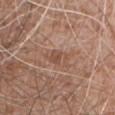Impression: The lesion was tiled from a total-body skin photograph and was not biopsied. Acquisition and patient details: The subject is a male about 60 years old. The tile uses white-light illumination. Located on the lower back. A lesion tile, about 15 mm wide, cut from a 3D total-body photograph. About 2.5 mm across. Automated tile analysis of the lesion measured an average lesion color of about L≈50 a*≈20 b*≈29 (CIELAB), a lesion–skin lightness drop of about 7, and a lesion-to-skin contrast of about 6 (normalized; higher = more distinct).